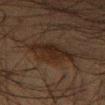Q: Was a biopsy performed?
A: no biopsy performed (imaged during a skin exam)
Q: How was this image acquired?
A: ~15 mm crop, total-body skin-cancer survey
Q: Illumination type?
A: cross-polarized
Q: What is the anatomic site?
A: the left thigh
Q: Patient demographics?
A: male, roughly 35 years of age
Q: How large is the lesion?
A: about 5.5 mm